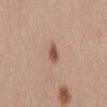{"biopsy_status": "not biopsied; imaged during a skin examination"}